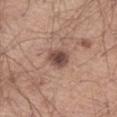Assessment:
The lesion was tiled from a total-body skin photograph and was not biopsied.
Acquisition and patient details:
Located on the left thigh. Imaged with white-light lighting. A male patient, aged 38–42. Cropped from a whole-body photographic skin survey; the tile spans about 15 mm.